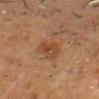Assessment: This lesion was catalogued during total-body skin photography and was not selected for biopsy. Image and clinical context: The lesion's longest dimension is about 3.5 mm. Cropped from a total-body skin-imaging series; the visible field is about 15 mm. On the head or neck. The patient is a male aged 48 to 52. Automated tile analysis of the lesion measured a footprint of about 6.5 mm² and an eccentricity of roughly 0.8. The software also gave roughly 7 lightness units darker than nearby skin. And it measured a nevus-likeness score of about 55/100 and lesion-presence confidence of about 100/100.